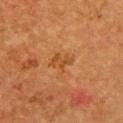{"biopsy_status": "not biopsied; imaged during a skin examination", "image": {"source": "total-body photography crop", "field_of_view_mm": 15}, "lighting": "cross-polarized", "patient": {"sex": "female", "age_approx": 50}, "site": "chest"}